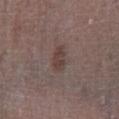Findings:
* notes: total-body-photography surveillance lesion; no biopsy
* site: the left lower leg
* image: ~15 mm tile from a whole-body skin photo
* subject: male, aged approximately 70